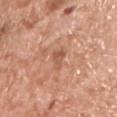Notes:
• notes · catalogued during a skin exam; not biopsied
• location · the chest
• lighting · white-light illumination
• patient · male, approximately 60 years of age
• TBP lesion metrics · a border-irregularity rating of about 4/10
• image source · 15 mm crop, total-body photography